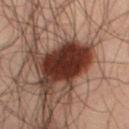This lesion was catalogued during total-body skin photography and was not selected for biopsy. This image is a 15 mm lesion crop taken from a total-body photograph. A male subject, about 50 years old. Located on the right thigh. Imaged with cross-polarized lighting.